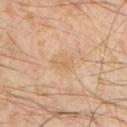Q: Is there a histopathology result?
A: total-body-photography surveillance lesion; no biopsy
Q: Where on the body is the lesion?
A: the chest
Q: What is the lesion's diameter?
A: about 3 mm
Q: What are the patient's age and sex?
A: male, about 30 years old
Q: How was this image acquired?
A: ~15 mm tile from a whole-body skin photo
Q: What lighting was used for the tile?
A: cross-polarized